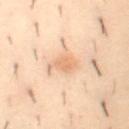A lesion tile, about 15 mm wide, cut from a 3D total-body photograph.
A male patient, roughly 30 years of age.
About 3.5 mm across.
On the abdomen.